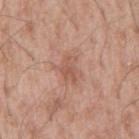Impression:
This lesion was catalogued during total-body skin photography and was not selected for biopsy.
Acquisition and patient details:
About 3 mm across. A male patient, aged 53 to 57. The lesion is located on the mid back. A 15 mm close-up extracted from a 3D total-body photography capture. An algorithmic analysis of the crop reported a mean CIELAB color near L≈55 a*≈22 b*≈29, about 8 CIELAB-L* units darker than the surrounding skin, and a lesion-to-skin contrast of about 5.5 (normalized; higher = more distinct). The analysis additionally found a border-irregularity rating of about 4/10 and peripheral color asymmetry of about 0.5. The analysis additionally found a nevus-likeness score of about 0/100.